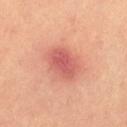Notes:
• image source — ~15 mm tile from a whole-body skin photo
• subject — female, approximately 50 years of age